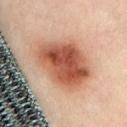Assessment: The lesion was photographed on a routine skin check and not biopsied; there is no pathology result. Acquisition and patient details: The subject is a female approximately 40 years of age. On the abdomen. Longest diameter approximately 7 mm. A region of skin cropped from a whole-body photographic capture, roughly 15 mm wide.